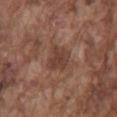site = the mid back; tile lighting = white-light; acquisition = ~15 mm tile from a whole-body skin photo; diameter = ≈3 mm; subject = male, aged approximately 75.